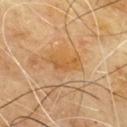Q: Is there a histopathology result?
A: no biopsy performed (imaged during a skin exam)
Q: What are the patient's age and sex?
A: male, roughly 65 years of age
Q: How was this image acquired?
A: ~15 mm crop, total-body skin-cancer survey
Q: How large is the lesion?
A: ≈4 mm
Q: What is the anatomic site?
A: the chest